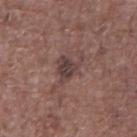biopsy status = no biopsy performed (imaged during a skin exam); image source = 15 mm crop, total-body photography; site = the front of the torso; lesion diameter = ~4 mm (longest diameter); patient = male, aged approximately 70.